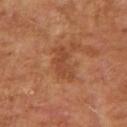Recorded during total-body skin imaging; not selected for excision or biopsy.